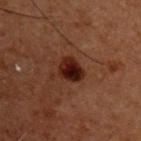lighting: cross-polarized
image:
  source: total-body photography crop
  field_of_view_mm: 15
site: chest
patient:
  sex: male
  age_approx: 60
lesion_size:
  long_diameter_mm_approx: 2.5